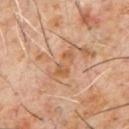Q: Was a biopsy performed?
A: total-body-photography surveillance lesion; no biopsy
Q: What did automated image analysis measure?
A: a footprint of about 6.5 mm², an eccentricity of roughly 0.9, and a shape-asymmetry score of about 0.35 (0 = symmetric); an average lesion color of about L≈54 a*≈20 b*≈34 (CIELAB) and roughly 7 lightness units darker than nearby skin; a border-irregularity rating of about 5/10, internal color variation of about 4 on a 0–10 scale, and a peripheral color-asymmetry measure near 1
Q: What are the patient's age and sex?
A: male, aged 58–62
Q: Lesion location?
A: the chest
Q: What kind of image is this?
A: ~15 mm crop, total-body skin-cancer survey
Q: Illumination type?
A: cross-polarized illumination
Q: What is the lesion's diameter?
A: about 4.5 mm